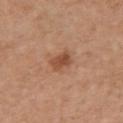biopsy status: total-body-photography surveillance lesion; no biopsy
subject: female, aged approximately 55
size: ≈2.5 mm
image source: total-body-photography crop, ~15 mm field of view
TBP lesion metrics: an average lesion color of about L≈49 a*≈23 b*≈32 (CIELAB), a lesion–skin lightness drop of about 11, and a lesion-to-skin contrast of about 7.5 (normalized; higher = more distinct); a nevus-likeness score of about 75/100 and a detector confidence of about 100 out of 100 that the crop contains a lesion
tile lighting: white-light
anatomic site: the front of the torso